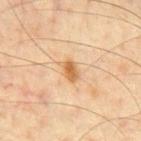The lesion was photographed on a routine skin check and not biopsied; there is no pathology result.
Imaged with cross-polarized lighting.
A roughly 15 mm field-of-view crop from a total-body skin photograph.
A male subject approximately 45 years of age.
Located on the chest.
Measured at roughly 2.5 mm in maximum diameter.